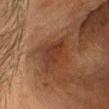<tbp_lesion>
<biopsy_status>not biopsied; imaged during a skin examination</biopsy_status>
<lesion_size>
  <long_diameter_mm_approx>4.5</long_diameter_mm_approx>
</lesion_size>
<image>
  <source>total-body photography crop</source>
  <field_of_view_mm>15</field_of_view_mm>
</image>
<site>head or neck</site>
<automated_metrics>
  <border_irregularity_0_10>3.0</border_irregularity_0_10>
  <color_variation_0_10>4.5</color_variation_0_10>
  <peripheral_color_asymmetry>1.5</peripheral_color_asymmetry>
  <lesion_detection_confidence_0_100>75</lesion_detection_confidence_0_100>
</automated_metrics>
<patient>
  <sex>male</sex>
  <age_approx>60</age_approx>
</patient>
</tbp_lesion>